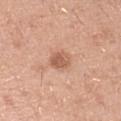Imaged during a routine full-body skin examination; the lesion was not biopsied and no histopathology is available. A male subject about 40 years old. The lesion's longest dimension is about 2.5 mm. A region of skin cropped from a whole-body photographic capture, roughly 15 mm wide. The lesion is located on the right upper arm. The tile uses white-light illumination. The lesion-visualizer software estimated an eccentricity of roughly 0.55.